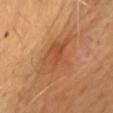biopsy status: catalogued during a skin exam; not biopsied | acquisition: ~15 mm tile from a whole-body skin photo | size: ~6 mm (longest diameter) | illumination: cross-polarized illumination | site: the chest | patient: male, roughly 65 years of age.